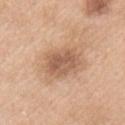Captured during whole-body skin photography for melanoma surveillance; the lesion was not biopsied.